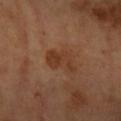| feature | finding |
|---|---|
| notes | catalogued during a skin exam; not biopsied |
| lesion size | ~4 mm (longest diameter) |
| subject | female, aged 58 to 62 |
| site | the right forearm |
| image source | total-body-photography crop, ~15 mm field of view |